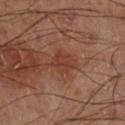Q: Is there a histopathology result?
A: imaged on a skin check; not biopsied
Q: How was this image acquired?
A: total-body-photography crop, ~15 mm field of view
Q: Illumination type?
A: cross-polarized
Q: What did automated image analysis measure?
A: a lesion area of about 6 mm², an outline eccentricity of about 0.65 (0 = round, 1 = elongated), and a shape-asymmetry score of about 0.35 (0 = symmetric); a lesion–skin lightness drop of about 7 and a normalized lesion–skin contrast near 6; internal color variation of about 2.5 on a 0–10 scale and radial color variation of about 1
Q: What is the lesion's diameter?
A: about 3 mm
Q: Lesion location?
A: the leg